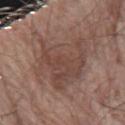Assessment:
Part of a total-body skin-imaging series; this lesion was reviewed on a skin check and was not flagged for biopsy.
Background:
The subject is a male aged around 80. A lesion tile, about 15 mm wide, cut from a 3D total-body photograph. The lesion's longest dimension is about 8.5 mm. On the right forearm. The tile uses white-light illumination.